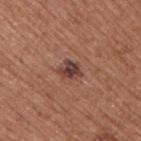• follow-up · imaged on a skin check; not biopsied
• imaging modality · ~15 mm crop, total-body skin-cancer survey
• automated lesion analysis · a footprint of about 4 mm² and two-axis asymmetry of about 0.3; an average lesion color of about L≈40 a*≈22 b*≈23 (CIELAB) and a normalized lesion–skin contrast near 10.5; a border-irregularity index near 3.5/10, a within-lesion color-variation index near 5/10, and peripheral color asymmetry of about 2; an automated nevus-likeness rating near 55 out of 100 and lesion-presence confidence of about 100/100
• subject · male, approximately 55 years of age
• site · the right upper arm